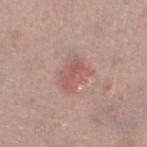A 15 mm close-up extracted from a 3D total-body photography capture. The tile uses white-light illumination. Approximately 3.5 mm at its widest. A female patient aged around 55. Located on the leg.